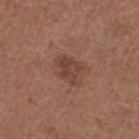Part of a total-body skin-imaging series; this lesion was reviewed on a skin check and was not flagged for biopsy. The recorded lesion diameter is about 3.5 mm. The lesion is on the left lower leg. A female patient, aged approximately 40. The lesion-visualizer software estimated an eccentricity of roughly 0.65 and a shape-asymmetry score of about 0.3 (0 = symmetric). It also reported border irregularity of about 3 on a 0–10 scale and a peripheral color-asymmetry measure near 1. This is a white-light tile. A 15 mm close-up tile from a total-body photography series done for melanoma screening.